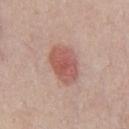• notes — catalogued during a skin exam; not biopsied
• lesion diameter — ≈4.5 mm
• subject — male, aged approximately 40
• tile lighting — white-light
• location — the chest
• image source — ~15 mm tile from a whole-body skin photo